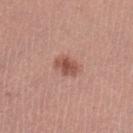<lesion>
<biopsy_status>not biopsied; imaged during a skin examination</biopsy_status>
<automated_metrics>
  <area_mm2_approx>5.0</area_mm2_approx>
  <shape_asymmetry>0.2</shape_asymmetry>
  <cielab_L>52</cielab_L>
  <cielab_a>24</cielab_a>
  <cielab_b>27</cielab_b>
  <vs_skin_darker_L>11.0</vs_skin_darker_L>
  <vs_skin_contrast_norm>8.0</vs_skin_contrast_norm>
  <border_irregularity_0_10>2.0</border_irregularity_0_10>
  <color_variation_0_10>3.0</color_variation_0_10>
  <peripheral_color_asymmetry>0.5</peripheral_color_asymmetry>
  <nevus_likeness_0_100>90</nevus_likeness_0_100>
  <lesion_detection_confidence_0_100>100</lesion_detection_confidence_0_100>
</automated_metrics>
<patient>
  <sex>female</sex>
  <age_approx>35</age_approx>
</patient>
<image>
  <source>total-body photography crop</source>
  <field_of_view_mm>15</field_of_view_mm>
</image>
<lesion_size>
  <long_diameter_mm_approx>3.0</long_diameter_mm_approx>
</lesion_size>
<site>left lower leg</site>
</lesion>